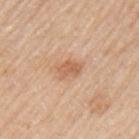Captured during whole-body skin photography for melanoma surveillance; the lesion was not biopsied.
A male subject, approximately 60 years of age.
Cropped from a whole-body photographic skin survey; the tile spans about 15 mm.
Located on the left upper arm.
Imaged with white-light lighting.
Approximately 3 mm at its widest.